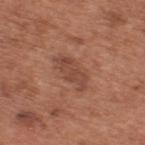Recorded during total-body skin imaging; not selected for excision or biopsy.
A 15 mm crop from a total-body photograph taken for skin-cancer surveillance.
The lesion is located on the upper back.
The patient is a male in their mid-60s.